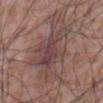{
  "biopsy_status": "not biopsied; imaged during a skin examination"
}